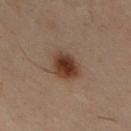image-analysis metrics: a shape eccentricity near 0.3 and two-axis asymmetry of about 0.15; a mean CIELAB color near L≈30 a*≈15 b*≈23, a lesion–skin lightness drop of about 10, and a lesion-to-skin contrast of about 10.5 (normalized; higher = more distinct); a border-irregularity index near 1.5/10, a within-lesion color-variation index near 4.5/10, and peripheral color asymmetry of about 1; an automated nevus-likeness rating near 100 out of 100 and a lesion-detection confidence of about 100/100
subject: male, aged around 30
imaging modality: total-body-photography crop, ~15 mm field of view
location: the chest
lesion diameter: about 3.5 mm